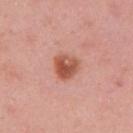Part of a total-body skin-imaging series; this lesion was reviewed on a skin check and was not flagged for biopsy.